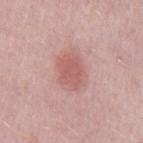Q: What did automated image analysis measure?
A: a normalized border contrast of about 6
Q: What is the imaging modality?
A: ~15 mm crop, total-body skin-cancer survey
Q: Where on the body is the lesion?
A: the left upper arm
Q: Who is the patient?
A: female, aged approximately 45
Q: Illumination type?
A: white-light